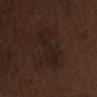Q: Was this lesion biopsied?
A: imaged on a skin check; not biopsied
Q: Illumination type?
A: white-light
Q: Lesion location?
A: the abdomen
Q: Lesion size?
A: about 6 mm
Q: How was this image acquired?
A: 15 mm crop, total-body photography
Q: What are the patient's age and sex?
A: male, aged 68–72